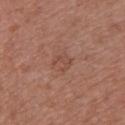The lesion was tiled from a total-body skin photograph and was not biopsied.
The lesion is located on the chest.
A close-up tile cropped from a whole-body skin photograph, about 15 mm across.
A female patient in their mid- to late 60s.